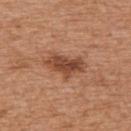Findings:
- workup: imaged on a skin check; not biopsied
- imaging modality: ~15 mm crop, total-body skin-cancer survey
- diameter: ~5 mm (longest diameter)
- subject: male, in their mid- to late 60s
- illumination: white-light illumination
- site: the upper back